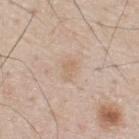biopsy status = catalogued during a skin exam; not biopsied
patient = male, about 75 years old
body site = the upper back
image source = total-body-photography crop, ~15 mm field of view